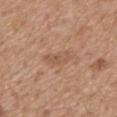follow-up: no biopsy performed (imaged during a skin exam) | image: total-body-photography crop, ~15 mm field of view | anatomic site: the mid back | lighting: white-light | TBP lesion metrics: a footprint of about 4.5 mm² and a symmetry-axis asymmetry near 0.35; an average lesion color of about L≈54 a*≈20 b*≈31 (CIELAB), roughly 7 lightness units darker than nearby skin, and a normalized border contrast of about 5; a color-variation rating of about 1.5/10 and a peripheral color-asymmetry measure near 0.5 | patient: male, about 70 years old.